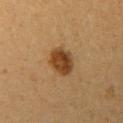Impression: Captured during whole-body skin photography for melanoma surveillance; the lesion was not biopsied. Clinical summary: About 4 mm across. The subject is a female aged 18–22. The lesion is located on the left upper arm. A 15 mm close-up tile from a total-body photography series done for melanoma screening. This is a cross-polarized tile.